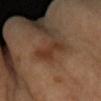Case summary:
- workup · total-body-photography surveillance lesion; no biopsy
- site · the right forearm
- imaging modality · ~15 mm crop, total-body skin-cancer survey
- diameter · ≈3.5 mm
- patient · female, roughly 60 years of age